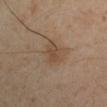follow-up: catalogued during a skin exam; not biopsied | body site: the arm | TBP lesion metrics: a footprint of about 4.5 mm², an eccentricity of roughly 0.75, and a symmetry-axis asymmetry near 0.25; an average lesion color of about L≈44 a*≈15 b*≈28 (CIELAB), a lesion–skin lightness drop of about 7, and a normalized border contrast of about 6; a within-lesion color-variation index near 3.5/10 and peripheral color asymmetry of about 1.5 | patient: male, roughly 40 years of age | image source: 15 mm crop, total-body photography | size: ~3 mm (longest diameter) | lighting: cross-polarized.